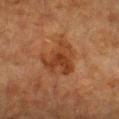Notes:
- workup · imaged on a skin check; not biopsied
- location · the chest
- image source · total-body-photography crop, ~15 mm field of view
- subject · female, in their mid- to late 50s
- illumination · cross-polarized illumination
- lesion diameter · ~4.5 mm (longest diameter)